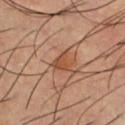Image and clinical context: This is a cross-polarized tile. Located on the chest. A male patient roughly 40 years of age. A roughly 15 mm field-of-view crop from a total-body skin photograph. The total-body-photography lesion software estimated a footprint of about 4.5 mm², an outline eccentricity of about 0.4 (0 = round, 1 = elongated), and a symmetry-axis asymmetry near 0.45.